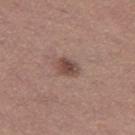{
  "biopsy_status": "not biopsied; imaged during a skin examination"
}